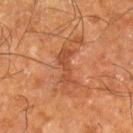{"biopsy_status": "not biopsied; imaged during a skin examination", "site": "leg", "patient": {"sex": "male", "age_approx": 65}, "image": {"source": "total-body photography crop", "field_of_view_mm": 15}}